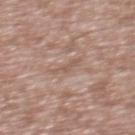notes: imaged on a skin check; not biopsied
site: the mid back
patient: male, aged approximately 45
imaging modality: 15 mm crop, total-body photography
lighting: white-light illumination